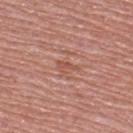workup: total-body-photography surveillance lesion; no biopsy | body site: the upper back | subject: male, aged 48 to 52 | lighting: white-light | TBP lesion metrics: a footprint of about 2.5 mm², a shape eccentricity near 0.9, and a shape-asymmetry score of about 0.45 (0 = symmetric); a mean CIELAB color near L≈53 a*≈25 b*≈29 and about 7 CIELAB-L* units darker than the surrounding skin | acquisition: ~15 mm tile from a whole-body skin photo.